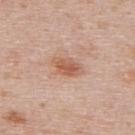notes: no biopsy performed (imaged during a skin exam); location: the upper back; acquisition: ~15 mm tile from a whole-body skin photo; patient: male, approximately 40 years of age; TBP lesion metrics: an average lesion color of about L≈58 a*≈23 b*≈31 (CIELAB); lighting: white-light illumination.